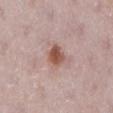Assessment: Imaged during a routine full-body skin examination; the lesion was not biopsied and no histopathology is available. Context: The patient is a female roughly 30 years of age. A lesion tile, about 15 mm wide, cut from a 3D total-body photograph. The lesion-visualizer software estimated two-axis asymmetry of about 0.25. The analysis additionally found an average lesion color of about L≈53 a*≈21 b*≈27 (CIELAB). Imaged with white-light lighting. The lesion is on the left lower leg.